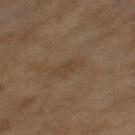Notes:
• workup · total-body-photography surveillance lesion; no biopsy
• lighting · cross-polarized
• subject · female, approximately 60 years of age
• acquisition · ~15 mm tile from a whole-body skin photo
• body site · the left thigh